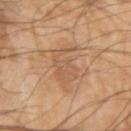Recorded during total-body skin imaging; not selected for excision or biopsy. The lesion-visualizer software estimated an automated nevus-likeness rating near 5 out of 100. Cropped from a whole-body photographic skin survey; the tile spans about 15 mm. Located on the arm. Approximately 6 mm at its widest. A male patient, roughly 65 years of age. Captured under cross-polarized illumination.